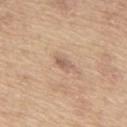{"biopsy_status": "not biopsied; imaged during a skin examination", "image": {"source": "total-body photography crop", "field_of_view_mm": 15}, "site": "upper back", "patient": {"sex": "male", "age_approx": 65}}